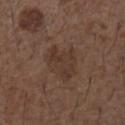Assessment:
This lesion was catalogued during total-body skin photography and was not selected for biopsy.
Clinical summary:
This image is a 15 mm lesion crop taken from a total-body photograph. On the arm. The patient is a male in their 50s. The lesion's longest dimension is about 5 mm. The tile uses white-light illumination.